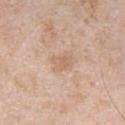Findings:
– workup — catalogued during a skin exam; not biopsied
– body site — the chest
– illumination — white-light
– acquisition — total-body-photography crop, ~15 mm field of view
– diameter — about 2.5 mm
– automated lesion analysis — an outline eccentricity of about 0.7 (0 = round, 1 = elongated) and two-axis asymmetry of about 0.2; a mean CIELAB color near L≈64 a*≈19 b*≈31, about 7 CIELAB-L* units darker than the surrounding skin, and a normalized border contrast of about 5
– subject — male, roughly 40 years of age